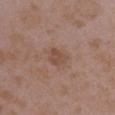This lesion was catalogued during total-body skin photography and was not selected for biopsy. A female subject aged around 35. A 15 mm close-up extracted from a 3D total-body photography capture. Longest diameter approximately 2.5 mm. From the left upper arm. Imaged with white-light lighting.